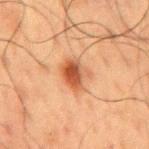Clinical impression: The lesion was photographed on a routine skin check and not biopsied; there is no pathology result. Acquisition and patient details: The lesion-visualizer software estimated a footprint of about 8 mm², a shape eccentricity near 0.65, and a symmetry-axis asymmetry near 0.25. And it measured a lesion color around L≈44 a*≈23 b*≈32 in CIELAB, roughly 11 lightness units darker than nearby skin, and a lesion-to-skin contrast of about 8.5 (normalized; higher = more distinct). And it measured a border-irregularity rating of about 2.5/10, internal color variation of about 6.5 on a 0–10 scale, and a peripheral color-asymmetry measure near 2. It also reported a nevus-likeness score of about 100/100. A 15 mm crop from a total-body photograph taken for skin-cancer surveillance. Longest diameter approximately 3.5 mm. The tile uses cross-polarized illumination. The subject is a male in their 60s. The lesion is on the back.